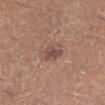Impression:
The lesion was photographed on a routine skin check and not biopsied; there is no pathology result.
Image and clinical context:
The patient is a male aged around 40. The lesion is located on the left lower leg. A roughly 15 mm field-of-view crop from a total-body skin photograph.